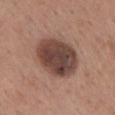Notes:
- biopsy status — catalogued during a skin exam; not biopsied
- subject — female, roughly 50 years of age
- image source — 15 mm crop, total-body photography
- site — the mid back